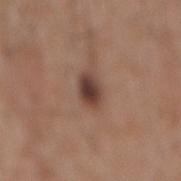  biopsy_status: not biopsied; imaged during a skin examination
  patient:
    sex: male
    age_approx: 60
  lighting: white-light
  lesion_size:
    long_diameter_mm_approx: 3.0
  automated_metrics:
    nevus_likeness_0_100: 95
    lesion_detection_confidence_0_100: 100
  image:
    source: total-body photography crop
    field_of_view_mm: 15
  site: mid back